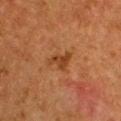notes: catalogued during a skin exam; not biopsied | location: the chest | illumination: cross-polarized | size: about 3 mm | subject: female, roughly 50 years of age | image source: ~15 mm crop, total-body skin-cancer survey.